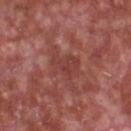workup=no biopsy performed (imaged during a skin exam) | site=the front of the torso | diameter=~4 mm (longest diameter) | automated lesion analysis=a border-irregularity rating of about 4/10, a within-lesion color-variation index near 2/10, and peripheral color asymmetry of about 0.5 | patient=male, aged 63 to 67 | image=15 mm crop, total-body photography | lighting=white-light.